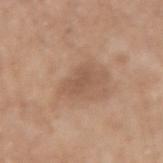workup = total-body-photography surveillance lesion; no biopsy
image-analysis metrics = an average lesion color of about L≈53 a*≈19 b*≈29 (CIELAB), a lesion–skin lightness drop of about 7, and a normalized border contrast of about 5
lesion size = ≈3 mm
site = the right upper arm
subject = male, aged 68–72
acquisition = total-body-photography crop, ~15 mm field of view